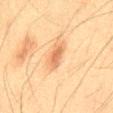Located on the front of the torso.
A male patient, about 35 years old.
An algorithmic analysis of the crop reported a mean CIELAB color near L≈53 a*≈20 b*≈33, about 9 CIELAB-L* units darker than the surrounding skin, and a lesion-to-skin contrast of about 7 (normalized; higher = more distinct). And it measured a color-variation rating of about 2.5/10 and radial color variation of about 0.5. The software also gave an automated nevus-likeness rating near 95 out of 100 and a lesion-detection confidence of about 100/100.
About 4 mm across.
Cropped from a total-body skin-imaging series; the visible field is about 15 mm.